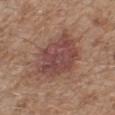workup: total-body-photography surveillance lesion; no biopsy
site: the leg
imaging modality: ~15 mm tile from a whole-body skin photo
patient: male, aged approximately 65
tile lighting: white-light illumination
automated metrics: a detector confidence of about 100 out of 100 that the crop contains a lesion